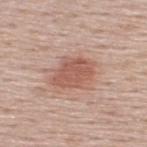– biopsy status · catalogued during a skin exam; not biopsied
– lesion diameter · about 5 mm
– image-analysis metrics · an area of roughly 13 mm², an eccentricity of roughly 0.7, and a symmetry-axis asymmetry near 0.2
– illumination · white-light illumination
– subject · male, aged 58–62
– image source · ~15 mm tile from a whole-body skin photo
– anatomic site · the upper back